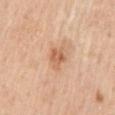- workup: no biopsy performed (imaged during a skin exam)
- lesion size: ~2.5 mm (longest diameter)
- image-analysis metrics: a footprint of about 4.5 mm² and a shape eccentricity near 0.5; an average lesion color of about L≈62 a*≈23 b*≈35 (CIELAB), roughly 10 lightness units darker than nearby skin, and a lesion-to-skin contrast of about 6.5 (normalized; higher = more distinct); a border-irregularity index near 3/10
- image source: 15 mm crop, total-body photography
- patient: male, about 50 years old
- anatomic site: the mid back